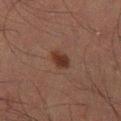The lesion was tiled from a total-body skin photograph and was not biopsied. A lesion tile, about 15 mm wide, cut from a 3D total-body photograph. On the right lower leg. The lesion's longest dimension is about 2.5 mm. The lesion-visualizer software estimated a lesion color around L≈25 a*≈17 b*≈21 in CIELAB, a lesion–skin lightness drop of about 8, and a lesion-to-skin contrast of about 9 (normalized; higher = more distinct). It also reported a border-irregularity index near 2/10 and internal color variation of about 1.5 on a 0–10 scale. Captured under cross-polarized illumination. A male subject aged approximately 50.